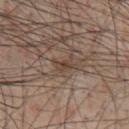biopsy status = total-body-photography surveillance lesion; no biopsy | image-analysis metrics = an eccentricity of roughly 0.8 and a symmetry-axis asymmetry near 0.45 | image source = 15 mm crop, total-body photography | tile lighting = white-light illumination | size = about 2.5 mm | site = the chest | patient = male, approximately 60 years of age.